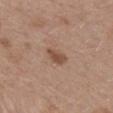| key | value |
|---|---|
| imaging modality | 15 mm crop, total-body photography |
| anatomic site | the mid back |
| patient | female, about 40 years old |